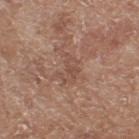Assessment: Recorded during total-body skin imaging; not selected for excision or biopsy. Acquisition and patient details: A close-up tile cropped from a whole-body skin photograph, about 15 mm across. On the right thigh. The lesion-visualizer software estimated a footprint of about 4.5 mm², an eccentricity of roughly 0.7, and a shape-asymmetry score of about 0.55 (0 = symmetric). The software also gave a peripheral color-asymmetry measure near 0.5. The analysis additionally found a nevus-likeness score of about 0/100 and a lesion-detection confidence of about 95/100. The patient is a female aged around 75.